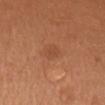{"biopsy_status": "not biopsied; imaged during a skin examination", "lighting": "white-light", "lesion_size": {"long_diameter_mm_approx": 2.5}, "automated_metrics": {"area_mm2_approx": 3.0, "eccentricity": 0.8, "shape_asymmetry": 0.35, "border_irregularity_0_10": 3.0, "color_variation_0_10": 1.0}, "site": "left upper arm", "patient": {"sex": "male", "age_approx": 65}, "image": {"source": "total-body photography crop", "field_of_view_mm": 15}}